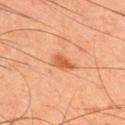Clinical impression:
Recorded during total-body skin imaging; not selected for excision or biopsy.
Acquisition and patient details:
Cropped from a total-body skin-imaging series; the visible field is about 15 mm. A male subject, approximately 45 years of age. On the back.